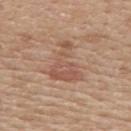Clinical impression:
This lesion was catalogued during total-body skin photography and was not selected for biopsy.
Clinical summary:
Cropped from a total-body skin-imaging series; the visible field is about 15 mm. A female patient, aged around 55. Located on the back.